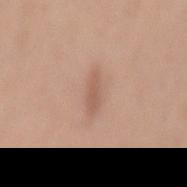<case>
<biopsy_status>not biopsied; imaged during a skin examination</biopsy_status>
<image>
  <source>total-body photography crop</source>
  <field_of_view_mm>15</field_of_view_mm>
</image>
<site>mid back</site>
<patient>
  <sex>female</sex>
  <age_approx>60</age_approx>
</patient>
<automated_metrics>
  <area_mm2_approx>3.5</area_mm2_approx>
  <eccentricity>0.95</eccentricity>
  <cielab_L>58</cielab_L>
  <cielab_a>20</cielab_a>
  <cielab_b>28</cielab_b>
  <vs_skin_darker_L>8.0</vs_skin_darker_L>
  <vs_skin_contrast_norm>5.5</vs_skin_contrast_norm>
  <border_irregularity_0_10>2.5</border_irregularity_0_10>
  <color_variation_0_10>1.0</color_variation_0_10>
  <peripheral_color_asymmetry>0.0</peripheral_color_asymmetry>
</automated_metrics>
<lesion_size>
  <long_diameter_mm_approx>3.5</long_diameter_mm_approx>
</lesion_size>
</case>